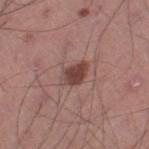notes: total-body-photography surveillance lesion; no biopsy | patient: male, aged 43 to 47 | illumination: white-light | anatomic site: the leg | diameter: about 3.5 mm | automated metrics: a lesion–skin lightness drop of about 12; a nevus-likeness score of about 85/100 and a detector confidence of about 100 out of 100 that the crop contains a lesion | imaging modality: ~15 mm tile from a whole-body skin photo.